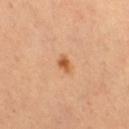A 15 mm close-up tile from a total-body photography series done for melanoma screening.
Measured at roughly 2 mm in maximum diameter.
Imaged with cross-polarized lighting.
The subject is a female about 55 years old.
Automated tile analysis of the lesion measured a lesion area of about 2.5 mm², an eccentricity of roughly 0.75, and a shape-asymmetry score of about 0.25 (0 = symmetric). The analysis additionally found a mean CIELAB color near L≈58 a*≈26 b*≈41, about 12 CIELAB-L* units darker than the surrounding skin, and a lesion-to-skin contrast of about 8.5 (normalized; higher = more distinct). The analysis additionally found border irregularity of about 2 on a 0–10 scale and peripheral color asymmetry of about 0.5. And it measured a classifier nevus-likeness of about 95/100 and a detector confidence of about 100 out of 100 that the crop contains a lesion.
Located on the right thigh.